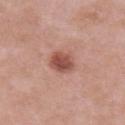This lesion was catalogued during total-body skin photography and was not selected for biopsy. A male subject, aged 53–57. This image is a 15 mm lesion crop taken from a total-body photograph. The lesion is on the right upper arm. The lesion's longest dimension is about 3 mm. Imaged with white-light lighting.